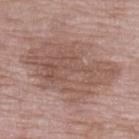notes = imaged on a skin check; not biopsied | acquisition = total-body-photography crop, ~15 mm field of view | lighting = white-light | location = the left lower leg | image-analysis metrics = roughly 9 lightness units darker than nearby skin and a normalized lesion–skin contrast near 6.5 | lesion size = ~10 mm (longest diameter) | subject = female, in their 70s.